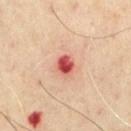A 15 mm close-up tile from a total-body photography series done for melanoma screening. A male subject, aged 68–72. Imaged with cross-polarized lighting. The lesion-visualizer software estimated a nevus-likeness score of about 0/100 and a detector confidence of about 100 out of 100 that the crop contains a lesion. From the chest. The lesion's longest dimension is about 2.5 mm.